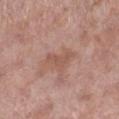- workup — no biopsy performed (imaged during a skin exam)